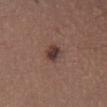| field | value |
|---|---|
| follow-up | catalogued during a skin exam; not biopsied |
| image | 15 mm crop, total-body photography |
| illumination | white-light |
| size | about 3 mm |
| automated lesion analysis | a footprint of about 4.5 mm², an outline eccentricity of about 0.7 (0 = round, 1 = elongated), and a symmetry-axis asymmetry near 0.3; border irregularity of about 2.5 on a 0–10 scale and a within-lesion color-variation index near 4.5/10; a nevus-likeness score of about 90/100 |
| site | the chest |
| subject | male, approximately 40 years of age |